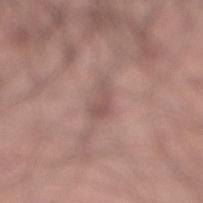The lesion was tiled from a total-body skin photograph and was not biopsied. Located on the right lower leg. A 15 mm crop from a total-body photograph taken for skin-cancer surveillance. A male subject aged 23 to 27. The recorded lesion diameter is about 3.5 mm.